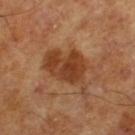Impression: The lesion was photographed on a routine skin check and not biopsied; there is no pathology result. Image and clinical context: A 15 mm crop from a total-body photograph taken for skin-cancer surveillance. The tile uses cross-polarized illumination. A male subject, about 65 years old. The lesion's longest dimension is about 6 mm. Automated image analysis of the tile measured a lesion color around L≈40 a*≈23 b*≈34 in CIELAB, roughly 10 lightness units darker than nearby skin, and a normalized lesion–skin contrast near 8.5. The analysis additionally found border irregularity of about 4 on a 0–10 scale, a within-lesion color-variation index near 3.5/10, and radial color variation of about 1.